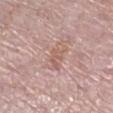Part of a total-body skin-imaging series; this lesion was reviewed on a skin check and was not flagged for biopsy. The total-body-photography lesion software estimated an area of roughly 4.5 mm², an eccentricity of roughly 0.85, and two-axis asymmetry of about 0.5. And it measured border irregularity of about 6.5 on a 0–10 scale, internal color variation of about 1 on a 0–10 scale, and a peripheral color-asymmetry measure near 0.5. The analysis additionally found an automated nevus-likeness rating near 0 out of 100 and a lesion-detection confidence of about 100/100. The lesion is on the right lower leg. A female subject roughly 60 years of age. This image is a 15 mm lesion crop taken from a total-body photograph.